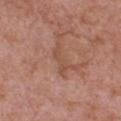subject=male, in their 60s
image=15 mm crop, total-body photography
lesion size=~3.5 mm (longest diameter)
location=the front of the torso
tile lighting=white-light